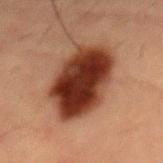Captured during whole-body skin photography for melanoma surveillance; the lesion was not biopsied. A 15 mm close-up extracted from a 3D total-body photography capture. The lesion is located on the abdomen. A male subject in their mid-50s.